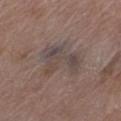Assessment:
The lesion was photographed on a routine skin check and not biopsied; there is no pathology result.
Context:
The tile uses white-light illumination. Automated image analysis of the tile measured a shape eccentricity near 0.75 and two-axis asymmetry of about 0.55. It also reported a lesion color around L≈44 a*≈12 b*≈18 in CIELAB. The software also gave border irregularity of about 7 on a 0–10 scale, a within-lesion color-variation index near 6/10, and a peripheral color-asymmetry measure near 2. It also reported an automated nevus-likeness rating near 0 out of 100 and a lesion-detection confidence of about 95/100. Cropped from a whole-body photographic skin survey; the tile spans about 15 mm. Located on the left thigh. The patient is a female approximately 70 years of age.